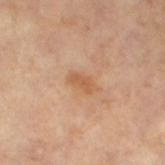follow-up=catalogued during a skin exam; not biopsied
location=the left lower leg
illumination=cross-polarized
image=total-body-photography crop, ~15 mm field of view
patient=male, aged approximately 60